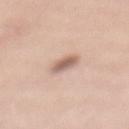The lesion is located on the mid back.
A female subject, aged around 35.
An algorithmic analysis of the crop reported a border-irregularity rating of about 2/10 and internal color variation of about 3.5 on a 0–10 scale. It also reported a nevus-likeness score of about 100/100 and a lesion-detection confidence of about 100/100.
A lesion tile, about 15 mm wide, cut from a 3D total-body photograph.